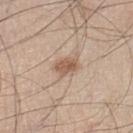biopsy status = catalogued during a skin exam; not biopsied | lesion diameter = about 3 mm | tile lighting = white-light illumination | image = 15 mm crop, total-body photography | site = the left lower leg | patient = male, aged approximately 45 | automated metrics = an area of roughly 5 mm², an eccentricity of roughly 0.7, and a symmetry-axis asymmetry near 0.2; a mean CIELAB color near L≈57 a*≈18 b*≈29 and a normalized lesion–skin contrast near 7.5; an automated nevus-likeness rating near 80 out of 100.